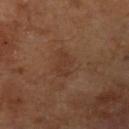Captured during whole-body skin photography for melanoma surveillance; the lesion was not biopsied.
A close-up tile cropped from a whole-body skin photograph, about 15 mm across.
A male subject, in their mid- to late 60s.
Longest diameter approximately 3.5 mm.
Imaged with cross-polarized lighting.
From the left upper arm.